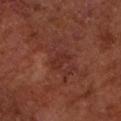The lesion was photographed on a routine skin check and not biopsied; there is no pathology result.
On the left forearm.
Cropped from a total-body skin-imaging series; the visible field is about 15 mm.
A male patient, aged 63 to 67.
An algorithmic analysis of the crop reported a lesion area of about 3.5 mm². The analysis additionally found a lesion color around L≈29 a*≈24 b*≈25 in CIELAB, roughly 5 lightness units darker than nearby skin, and a lesion-to-skin contrast of about 5 (normalized; higher = more distinct). It also reported an automated nevus-likeness rating near 0 out of 100.
This is a cross-polarized tile.
Measured at roughly 3 mm in maximum diameter.